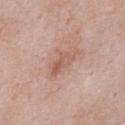Assessment:
Part of a total-body skin-imaging series; this lesion was reviewed on a skin check and was not flagged for biopsy.
Context:
On the abdomen. A male patient, approximately 55 years of age. Longest diameter approximately 3.5 mm. Captured under white-light illumination. Automated image analysis of the tile measured a normalized lesion–skin contrast near 6. A 15 mm close-up extracted from a 3D total-body photography capture.